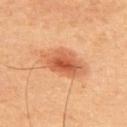lesion diameter: ~6 mm (longest diameter) | subject: male, aged around 60 | tile lighting: cross-polarized illumination | image: ~15 mm crop, total-body skin-cancer survey | automated lesion analysis: a lesion color around L≈58 a*≈28 b*≈38 in CIELAB, roughly 12 lightness units darker than nearby skin, and a normalized border contrast of about 7.5; border irregularity of about 2.5 on a 0–10 scale, a color-variation rating of about 6.5/10, and a peripheral color-asymmetry measure near 2 | site: the upper back.